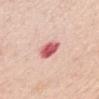<record>
<biopsy_status>not biopsied; imaged during a skin examination</biopsy_status>
<patient>
  <sex>female</sex>
  <age_approx>65</age_approx>
</patient>
<image>
  <source>total-body photography crop</source>
  <field_of_view_mm>15</field_of_view_mm>
</image>
<lighting>white-light</lighting>
<site>front of the torso</site>
<automated_metrics>
  <nevus_likeness_0_100>0</nevus_likeness_0_100>
  <lesion_detection_confidence_0_100>100</lesion_detection_confidence_0_100>
</automated_metrics>
</record>